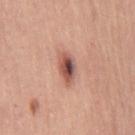Impression:
The lesion was photographed on a routine skin check and not biopsied; there is no pathology result.
Context:
A female subject, aged 48–52. The lesion's longest dimension is about 4 mm. This is a white-light tile. A lesion tile, about 15 mm wide, cut from a 3D total-body photograph. The lesion is located on the leg.